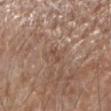<case>
  <biopsy_status>not biopsied; imaged during a skin examination</biopsy_status>
  <image>
    <source>total-body photography crop</source>
    <field_of_view_mm>15</field_of_view_mm>
  </image>
  <site>left forearm</site>
  <lighting>white-light</lighting>
  <patient>
    <sex>male</sex>
    <age_approx>75</age_approx>
  </patient>
</case>